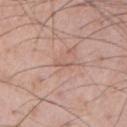<case>
<biopsy_status>not biopsied; imaged during a skin examination</biopsy_status>
<patient>
  <sex>male</sex>
  <age_approx>60</age_approx>
</patient>
<automated_metrics>
  <area_mm2_approx>2.0</area_mm2_approx>
  <eccentricity>0.95</eccentricity>
  <shape_asymmetry>0.35</shape_asymmetry>
  <vs_skin_darker_L>7.0</vs_skin_darker_L>
  <vs_skin_contrast_norm>4.5</vs_skin_contrast_norm>
  <border_irregularity_0_10>4.0</border_irregularity_0_10>
  <color_variation_0_10>0.0</color_variation_0_10>
  <peripheral_color_asymmetry>0.0</peripheral_color_asymmetry>
  <nevus_likeness_0_100>0</nevus_likeness_0_100>
  <lesion_detection_confidence_0_100>95</lesion_detection_confidence_0_100>
</automated_metrics>
<image>
  <source>total-body photography crop</source>
  <field_of_view_mm>15</field_of_view_mm>
</image>
<site>chest</site>
<lesion_size>
  <long_diameter_mm_approx>2.5</long_diameter_mm_approx>
</lesion_size>
</case>